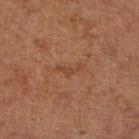Q: Was this lesion biopsied?
A: total-body-photography surveillance lesion; no biopsy
Q: Illumination type?
A: cross-polarized illumination
Q: What is the imaging modality?
A: total-body-photography crop, ~15 mm field of view
Q: Who is the patient?
A: male, in their 60s
Q: What did automated image analysis measure?
A: a shape eccentricity near 0.9 and a symmetry-axis asymmetry near 0.6; a classifier nevus-likeness of about 0/100 and lesion-presence confidence of about 100/100
Q: What is the anatomic site?
A: the leg
Q: Lesion size?
A: about 3 mm